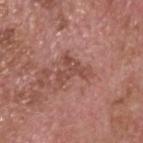Part of a total-body skin-imaging series; this lesion was reviewed on a skin check and was not flagged for biopsy.